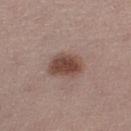  biopsy_status: not biopsied; imaged during a skin examination
  lighting: white-light
  image:
    source: total-body photography crop
    field_of_view_mm: 15
  site: left thigh
  patient:
    sex: female
    age_approx: 35